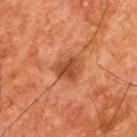Imaged during a routine full-body skin examination; the lesion was not biopsied and no histopathology is available.
Cropped from a total-body skin-imaging series; the visible field is about 15 mm.
Automated tile analysis of the lesion measured a lesion area of about 7 mm², an eccentricity of roughly 0.55, and a symmetry-axis asymmetry near 0.25. And it measured a classifier nevus-likeness of about 10/100 and a detector confidence of about 100 out of 100 that the crop contains a lesion.
The lesion's longest dimension is about 3 mm.
A male subject in their mid- to late 60s.
On the back.
This is a cross-polarized tile.